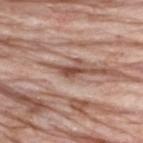<lesion>
<biopsy_status>not biopsied; imaged during a skin examination</biopsy_status>
<patient>
  <sex>male</sex>
  <age_approx>60</age_approx>
</patient>
<automated_metrics>
  <area_mm2_approx>2.5</area_mm2_approx>
  <border_irregularity_0_10>4.5</border_irregularity_0_10>
  <color_variation_0_10>0.0</color_variation_0_10>
  <peripheral_color_asymmetry>0.0</peripheral_color_asymmetry>
</automated_metrics>
<site>upper back</site>
<lesion_size>
  <long_diameter_mm_approx>2.5</long_diameter_mm_approx>
</lesion_size>
<image>
  <source>total-body photography crop</source>
  <field_of_view_mm>15</field_of_view_mm>
</image>
<lighting>white-light</lighting>
</lesion>